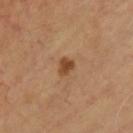| key | value |
|---|---|
| follow-up | catalogued during a skin exam; not biopsied |
| subject | male, about 55 years old |
| lesion diameter | ≈2.5 mm |
| tile lighting | cross-polarized illumination |
| body site | the front of the torso |
| imaging modality | 15 mm crop, total-body photography |
| TBP lesion metrics | a footprint of about 3.5 mm² and an eccentricity of roughly 0.55; an average lesion color of about L≈45 a*≈21 b*≈35 (CIELAB), a lesion–skin lightness drop of about 11, and a normalized lesion–skin contrast near 8.5; a color-variation rating of about 2.5/10 |